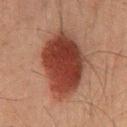Part of a total-body skin-imaging series; this lesion was reviewed on a skin check and was not flagged for biopsy. A male subject, in their 60s. The lesion's longest dimension is about 8.5 mm. Imaged with cross-polarized lighting. From the front of the torso. A 15 mm crop from a total-body photograph taken for skin-cancer surveillance.